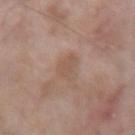Case summary:
– biopsy status: imaged on a skin check; not biopsied
– anatomic site: the arm
– lesion diameter: about 3 mm
– subject: male, aged around 60
– image: 15 mm crop, total-body photography